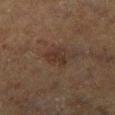Clinical impression:
Captured during whole-body skin photography for melanoma surveillance; the lesion was not biopsied.
Clinical summary:
Located on the leg. The total-body-photography lesion software estimated a footprint of about 5 mm², an outline eccentricity of about 0.7 (0 = round, 1 = elongated), and a shape-asymmetry score of about 0.45 (0 = symmetric). A lesion tile, about 15 mm wide, cut from a 3D total-body photograph. The patient is a male aged around 75. Measured at roughly 3 mm in maximum diameter. The tile uses cross-polarized illumination.